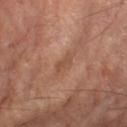patient = male, about 65 years old; anatomic site = the left lower leg; tile lighting = cross-polarized; acquisition = total-body-photography crop, ~15 mm field of view; size = ~3 mm (longest diameter).